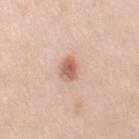This lesion was catalogued during total-body skin photography and was not selected for biopsy. A region of skin cropped from a whole-body photographic capture, roughly 15 mm wide. Automated image analysis of the tile measured an average lesion color of about L≈62 a*≈22 b*≈30 (CIELAB), roughly 13 lightness units darker than nearby skin, and a normalized border contrast of about 8. Approximately 3 mm at its widest. The lesion is on the arm. A female patient, about 40 years old.